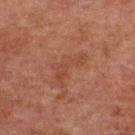Imaged during a routine full-body skin examination; the lesion was not biopsied and no histopathology is available.
A roughly 15 mm field-of-view crop from a total-body skin photograph.
The total-body-photography lesion software estimated a footprint of about 7.5 mm², a shape eccentricity near 0.85, and a symmetry-axis asymmetry near 0.65. And it measured an average lesion color of about L≈34 a*≈19 b*≈25 (CIELAB), a lesion–skin lightness drop of about 4, and a normalized border contrast of about 4.5.
The patient is a male about 60 years old.
The tile uses cross-polarized illumination.
The lesion is located on the upper back.
About 4.5 mm across.